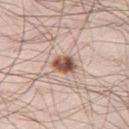Impression:
Recorded during total-body skin imaging; not selected for excision or biopsy.
Background:
A 15 mm crop from a total-body photograph taken for skin-cancer surveillance. Approximately 3 mm at its widest. Imaged with white-light lighting. A male subject aged approximately 35. The total-body-photography lesion software estimated an outline eccentricity of about 0.65 (0 = round, 1 = elongated) and a shape-asymmetry score of about 0.2 (0 = symmetric). The analysis additionally found a mean CIELAB color near L≈53 a*≈20 b*≈27, roughly 17 lightness units darker than nearby skin, and a normalized border contrast of about 11.5. And it measured a border-irregularity rating of about 1.5/10, a color-variation rating of about 7.5/10, and a peripheral color-asymmetry measure near 3. And it measured a lesion-detection confidence of about 100/100. Located on the right thigh.